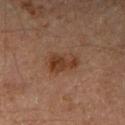Q: What are the patient's age and sex?
A: male, aged around 45
Q: How was this image acquired?
A: 15 mm crop, total-body photography
Q: Lesion location?
A: the right upper arm
Q: Lesion size?
A: ~4 mm (longest diameter)
Q: Automated lesion metrics?
A: a border-irregularity rating of about 2.5/10, a within-lesion color-variation index near 4.5/10, and peripheral color asymmetry of about 1.5
Q: How was the tile lit?
A: cross-polarized illumination